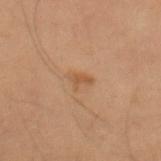Q: Was a biopsy performed?
A: total-body-photography surveillance lesion; no biopsy
Q: How was this image acquired?
A: ~15 mm crop, total-body skin-cancer survey
Q: Patient demographics?
A: male, about 50 years old
Q: How large is the lesion?
A: ≈2 mm
Q: What lighting was used for the tile?
A: cross-polarized illumination
Q: What did automated image analysis measure?
A: an area of roughly 2 mm², a shape eccentricity near 0.8, and two-axis asymmetry of about 0.4; a color-variation rating of about 0/10; an automated nevus-likeness rating near 5 out of 100 and a detector confidence of about 100 out of 100 that the crop contains a lesion
Q: Lesion location?
A: the arm